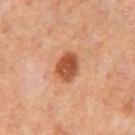<tbp_lesion>
<biopsy_status>not biopsied; imaged during a skin examination</biopsy_status>
<patient>
  <sex>female</sex>
  <age_approx>50</age_approx>
</patient>
<image>
  <source>total-body photography crop</source>
  <field_of_view_mm>15</field_of_view_mm>
</image>
<lighting>cross-polarized</lighting>
<site>leg</site>
<lesion_size>
  <long_diameter_mm_approx>4.0</long_diameter_mm_approx>
</lesion_size>
</tbp_lesion>